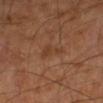Clinical summary: A male subject, in their 60s. The tile uses cross-polarized illumination. On the left forearm. The recorded lesion diameter is about 3.5 mm. Automated tile analysis of the lesion measured border irregularity of about 5.5 on a 0–10 scale, internal color variation of about 1 on a 0–10 scale, and peripheral color asymmetry of about 0.5. And it measured an automated nevus-likeness rating near 0 out of 100 and a detector confidence of about 100 out of 100 that the crop contains a lesion. A 15 mm close-up tile from a total-body photography series done for melanoma screening.